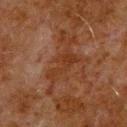Clinical impression:
Captured during whole-body skin photography for melanoma surveillance; the lesion was not biopsied.
Context:
The lesion-visualizer software estimated a footprint of about 5.5 mm² and a shape eccentricity near 0.9. It also reported an average lesion color of about L≈27 a*≈20 b*≈27 (CIELAB) and roughly 5 lightness units darker than nearby skin. It also reported border irregularity of about 8.5 on a 0–10 scale, a within-lesion color-variation index near 1/10, and peripheral color asymmetry of about 0.5. The software also gave a classifier nevus-likeness of about 0/100 and a lesion-detection confidence of about 90/100. Located on the upper back. Imaged with cross-polarized lighting. The subject is a male about 80 years old. Longest diameter approximately 4.5 mm. A region of skin cropped from a whole-body photographic capture, roughly 15 mm wide.